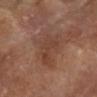Impression: The lesion was photographed on a routine skin check and not biopsied; there is no pathology result. Clinical summary: Located on the left upper arm. A female patient, aged 73–77. Cropped from a whole-body photographic skin survey; the tile spans about 15 mm.